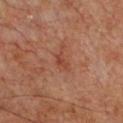| feature | finding |
|---|---|
| workup | no biopsy performed (imaged during a skin exam) |
| size | about 2.5 mm |
| site | the upper back |
| patient | male, in their 60s |
| tile lighting | cross-polarized illumination |
| image | ~15 mm tile from a whole-body skin photo |